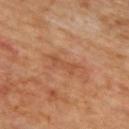Q: Is there a histopathology result?
A: total-body-photography surveillance lesion; no biopsy
Q: Patient demographics?
A: male, aged approximately 70
Q: What is the lesion's diameter?
A: about 3.5 mm
Q: What lighting was used for the tile?
A: cross-polarized
Q: Where on the body is the lesion?
A: the upper back
Q: What kind of image is this?
A: ~15 mm crop, total-body skin-cancer survey